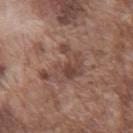Recorded during total-body skin imaging; not selected for excision or biopsy. The lesion is on the front of the torso. Automated image analysis of the tile measured a footprint of about 12 mm², an eccentricity of roughly 0.65, and a symmetry-axis asymmetry near 0.6. The analysis additionally found an automated nevus-likeness rating near 0 out of 100 and lesion-presence confidence of about 100/100. A 15 mm close-up extracted from a 3D total-body photography capture. A male subject, aged 73–77. The lesion's longest dimension is about 5 mm.